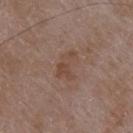Imaged during a routine full-body skin examination; the lesion was not biopsied and no histopathology is available. A female subject roughly 70 years of age. The lesion-visualizer software estimated a lesion area of about 5.5 mm² and an eccentricity of roughly 0.85. The software also gave a mean CIELAB color near L≈45 a*≈18 b*≈26 and a lesion-to-skin contrast of about 5.5 (normalized; higher = more distinct). It also reported a border-irregularity index near 6/10. Imaged with white-light lighting. Cropped from a total-body skin-imaging series; the visible field is about 15 mm. Longest diameter approximately 3.5 mm. On the upper back.